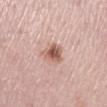biopsy_status: not biopsied; imaged during a skin examination
patient:
  sex: female
  age_approx: 40
site: left lower leg
image:
  source: total-body photography crop
  field_of_view_mm: 15